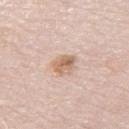• notes: no biopsy performed (imaged during a skin exam)
• patient: male, aged 78–82
• location: the left thigh
• image: ~15 mm tile from a whole-body skin photo